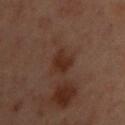Findings:
* patient · female, about 55 years old
* anatomic site · the left upper arm
* lesion diameter · about 2.5 mm
* acquisition · total-body-photography crop, ~15 mm field of view